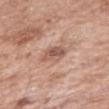The lesion was tiled from a total-body skin photograph and was not biopsied.
A region of skin cropped from a whole-body photographic capture, roughly 15 mm wide.
A female subject aged 68–72.
The lesion is located on the right upper arm.
About 3 mm across.
The tile uses white-light illumination.